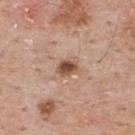Captured during whole-body skin photography for melanoma surveillance; the lesion was not biopsied. A region of skin cropped from a whole-body photographic capture, roughly 15 mm wide. A male patient aged approximately 60. The lesion is located on the upper back. Captured under white-light illumination.